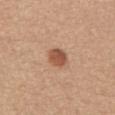notes: imaged on a skin check; not biopsied | patient: female, aged around 55 | size: ~2.5 mm (longest diameter) | image: total-body-photography crop, ~15 mm field of view | site: the abdomen | illumination: white-light illumination.